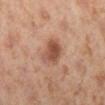Assessment:
Part of a total-body skin-imaging series; this lesion was reviewed on a skin check and was not flagged for biopsy.
Context:
Automated tile analysis of the lesion measured a lesion area of about 7 mm² and a shape eccentricity near 0.75. The software also gave a mean CIELAB color near L≈50 a*≈23 b*≈30, about 12 CIELAB-L* units darker than the surrounding skin, and a normalized lesion–skin contrast near 8.5. And it measured an automated nevus-likeness rating near 80 out of 100 and a detector confidence of about 100 out of 100 that the crop contains a lesion. The lesion is on the right lower leg. A female patient, about 40 years old. A 15 mm crop from a total-body photograph taken for skin-cancer surveillance. About 3.5 mm across.